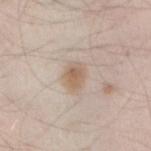Imaged during a routine full-body skin examination; the lesion was not biopsied and no histopathology is available.
This is a white-light tile.
Located on the right forearm.
Measured at roughly 3 mm in maximum diameter.
The subject is a male roughly 45 years of age.
The total-body-photography lesion software estimated an area of roughly 6 mm² and an outline eccentricity of about 0.7 (0 = round, 1 = elongated). The analysis additionally found a nevus-likeness score of about 95/100 and a detector confidence of about 100 out of 100 that the crop contains a lesion.
Cropped from a total-body skin-imaging series; the visible field is about 15 mm.